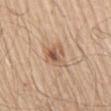Impression:
Imaged during a routine full-body skin examination; the lesion was not biopsied and no histopathology is available.
Acquisition and patient details:
The recorded lesion diameter is about 3.5 mm. This is a white-light tile. The subject is a male about 70 years old. Automated tile analysis of the lesion measured a shape eccentricity near 0.65 and two-axis asymmetry of about 0.35. The analysis additionally found a mean CIELAB color near L≈58 a*≈19 b*≈31, roughly 10 lightness units darker than nearby skin, and a normalized lesion–skin contrast near 7. It also reported a border-irregularity index near 3.5/10, a within-lesion color-variation index near 8.5/10, and a peripheral color-asymmetry measure near 3. It also reported a nevus-likeness score of about 55/100. A lesion tile, about 15 mm wide, cut from a 3D total-body photograph. Located on the right upper arm.